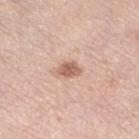The lesion was photographed on a routine skin check and not biopsied; there is no pathology result. Imaged with white-light lighting. Located on the left lower leg. A female subject aged 63–67. The recorded lesion diameter is about 2.5 mm. A 15 mm close-up tile from a total-body photography series done for melanoma screening.